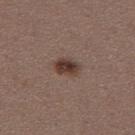No biopsy was performed on this lesion — it was imaged during a full skin examination and was not determined to be concerning. A close-up tile cropped from a whole-body skin photograph, about 15 mm across. Automated image analysis of the tile measured a footprint of about 5.5 mm² and a shape eccentricity near 0.7. The analysis additionally found a lesion-to-skin contrast of about 10 (normalized; higher = more distinct). On the left thigh. Imaged with white-light lighting. The lesion's longest dimension is about 3 mm. A female subject roughly 30 years of age.